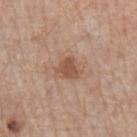Clinical impression:
This lesion was catalogued during total-body skin photography and was not selected for biopsy.
Acquisition and patient details:
The lesion is on the left forearm. A male patient, aged around 60. Captured under white-light illumination. The lesion's longest dimension is about 3 mm. Cropped from a total-body skin-imaging series; the visible field is about 15 mm.